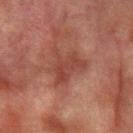Findings:
– subject · male, roughly 75 years of age
– location · the left forearm
– imaging modality · total-body-photography crop, ~15 mm field of view
– TBP lesion metrics · a lesion color around L≈35 a*≈23 b*≈24 in CIELAB, about 7 CIELAB-L* units darker than the surrounding skin, and a lesion-to-skin contrast of about 6.5 (normalized; higher = more distinct); border irregularity of about 6.5 on a 0–10 scale, internal color variation of about 2.5 on a 0–10 scale, and radial color variation of about 1